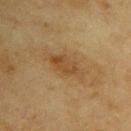This lesion was catalogued during total-body skin photography and was not selected for biopsy. On the right upper arm. Automated image analysis of the tile measured an average lesion color of about L≈41 a*≈16 b*≈33 (CIELAB). The software also gave a border-irregularity rating of about 3/10 and a peripheral color-asymmetry measure near 1. And it measured a nevus-likeness score of about 0/100 and lesion-presence confidence of about 100/100. Captured under cross-polarized illumination. This image is a 15 mm lesion crop taken from a total-body photograph. The subject is a female aged 53 to 57.